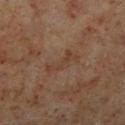workup — total-body-photography surveillance lesion; no biopsy | patient — male, aged approximately 60 | size — about 4.5 mm | imaging modality — ~15 mm crop, total-body skin-cancer survey | location — the right lower leg | illumination — cross-polarized.